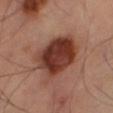Assessment: The lesion was tiled from a total-body skin photograph and was not biopsied. Context: Captured under cross-polarized illumination. A 15 mm close-up extracted from a 3D total-body photography capture. On the right thigh.